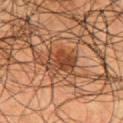| feature | finding |
|---|---|
| workup | total-body-photography surveillance lesion; no biopsy |
| tile lighting | cross-polarized |
| automated lesion analysis | border irregularity of about 3.5 on a 0–10 scale, a within-lesion color-variation index near 5/10, and peripheral color asymmetry of about 2; an automated nevus-likeness rating near 70 out of 100 and a lesion-detection confidence of about 95/100 |
| subject | male, aged around 55 |
| acquisition | ~15 mm tile from a whole-body skin photo |
| anatomic site | the chest |
| lesion size | ~3.5 mm (longest diameter) |